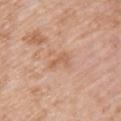Q: What is the anatomic site?
A: the left upper arm
Q: Lesion size?
A: ≈3 mm
Q: How was this image acquired?
A: total-body-photography crop, ~15 mm field of view
Q: Illumination type?
A: white-light illumination
Q: Patient demographics?
A: female, in their 70s